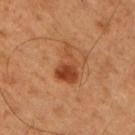Q: Was a biopsy performed?
A: total-body-photography surveillance lesion; no biopsy
Q: How was the tile lit?
A: cross-polarized
Q: Lesion location?
A: the right upper arm
Q: Patient demographics?
A: male, aged around 50
Q: What kind of image is this?
A: ~15 mm tile from a whole-body skin photo